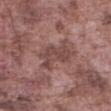No biopsy was performed on this lesion — it was imaged during a full skin examination and was not determined to be concerning.
Imaged with white-light lighting.
A 15 mm crop from a total-body photograph taken for skin-cancer surveillance.
Automated image analysis of the tile measured a lesion area of about 10 mm². The software also gave an average lesion color of about L≈45 a*≈20 b*≈21 (CIELAB), roughly 9 lightness units darker than nearby skin, and a normalized border contrast of about 6.5. And it measured a border-irregularity index near 5/10, a within-lesion color-variation index near 2.5/10, and peripheral color asymmetry of about 1. And it measured an automated nevus-likeness rating near 0 out of 100 and a lesion-detection confidence of about 85/100.
The lesion is located on the abdomen.
A male patient aged around 75.
Approximately 5 mm at its widest.